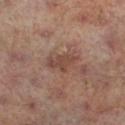follow-up — catalogued during a skin exam; not biopsied | site — the right lower leg | automated lesion analysis — a footprint of about 7 mm², a shape eccentricity near 0.85, and two-axis asymmetry of about 0.25; a lesion color around L≈43 a*≈19 b*≈24 in CIELAB and a normalized border contrast of about 6.5; border irregularity of about 3.5 on a 0–10 scale, internal color variation of about 2.5 on a 0–10 scale, and a peripheral color-asymmetry measure near 1; a lesion-detection confidence of about 100/100 | patient — male, about 65 years old | image — ~15 mm crop, total-body skin-cancer survey | lesion size — about 4 mm | lighting — cross-polarized illumination.